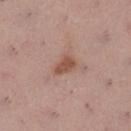{
  "biopsy_status": "not biopsied; imaged during a skin examination",
  "site": "left lower leg",
  "automated_metrics": {
    "area_mm2_approx": 5.0,
    "eccentricity": 0.75,
    "shape_asymmetry": 0.35
  },
  "patient": {
    "sex": "female",
    "age_approx": 55
  },
  "image": {
    "source": "total-body photography crop",
    "field_of_view_mm": 15
  },
  "lesion_size": {
    "long_diameter_mm_approx": 3.0
  }
}